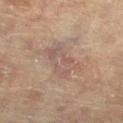Findings:
- notes — imaged on a skin check; not biopsied
- tile lighting — cross-polarized
- image source — ~15 mm tile from a whole-body skin photo
- patient — female, in their 80s
- automated lesion analysis — an automated nevus-likeness rating near 0 out of 100 and a detector confidence of about 80 out of 100 that the crop contains a lesion
- body site — the right lower leg
- size — about 5 mm